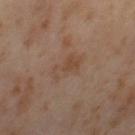Impression:
The lesion was tiled from a total-body skin photograph and was not biopsied.
Acquisition and patient details:
A female subject, aged around 55. A 15 mm close-up tile from a total-body photography series done for melanoma screening. Captured under cross-polarized illumination. Automated tile analysis of the lesion measured an outline eccentricity of about 0.85 (0 = round, 1 = elongated). The analysis additionally found a border-irregularity rating of about 3.5/10, a color-variation rating of about 4/10, and a peripheral color-asymmetry measure near 1.5. From the left thigh.